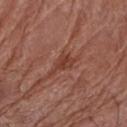The lesion was tiled from a total-body skin photograph and was not biopsied.
Captured under white-light illumination.
The total-body-photography lesion software estimated a footprint of about 5 mm², an outline eccentricity of about 0.85 (0 = round, 1 = elongated), and two-axis asymmetry of about 0.55. And it measured a mean CIELAB color near L≈41 a*≈25 b*≈28, about 8 CIELAB-L* units darker than the surrounding skin, and a normalized border contrast of about 6.5. And it measured internal color variation of about 3 on a 0–10 scale and radial color variation of about 1.
The lesion's longest dimension is about 4 mm.
The lesion is located on the right forearm.
Cropped from a total-body skin-imaging series; the visible field is about 15 mm.
A female subject aged around 80.